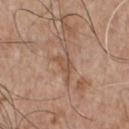Impression: This lesion was catalogued during total-body skin photography and was not selected for biopsy. Acquisition and patient details: The recorded lesion diameter is about 2.5 mm. Located on the chest. A male subject, roughly 65 years of age. Captured under white-light illumination. A close-up tile cropped from a whole-body skin photograph, about 15 mm across.